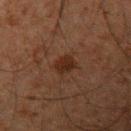Q: Was this lesion biopsied?
A: imaged on a skin check; not biopsied
Q: What is the anatomic site?
A: the left upper arm
Q: What is the imaging modality?
A: 15 mm crop, total-body photography
Q: What are the patient's age and sex?
A: male, approximately 35 years of age